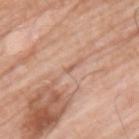Captured during whole-body skin photography for melanoma surveillance; the lesion was not biopsied.
Cropped from a whole-body photographic skin survey; the tile spans about 15 mm.
The lesion is on the upper back.
A male subject, aged around 65.